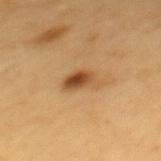This lesion was catalogued during total-body skin photography and was not selected for biopsy. The recorded lesion diameter is about 3.5 mm. Automated image analysis of the tile measured a mean CIELAB color near L≈50 a*≈21 b*≈39, about 12 CIELAB-L* units darker than the surrounding skin, and a normalized lesion–skin contrast near 9. The patient is a male aged 58 to 62. On the mid back. This is a cross-polarized tile. A lesion tile, about 15 mm wide, cut from a 3D total-body photograph.